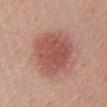Context:
A lesion tile, about 15 mm wide, cut from a 3D total-body photograph. Automated tile analysis of the lesion measured border irregularity of about 2 on a 0–10 scale, a within-lesion color-variation index near 3/10, and peripheral color asymmetry of about 1. And it measured a classifier nevus-likeness of about 100/100. The subject is a male roughly 65 years of age. This is a white-light tile. The lesion is located on the front of the torso. Approximately 6.5 mm at its widest.